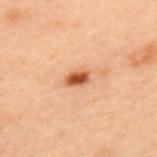Recorded during total-body skin imaging; not selected for excision or biopsy. The subject is a male aged approximately 55. Approximately 2.5 mm at its widest. A 15 mm crop from a total-body photograph taken for skin-cancer surveillance. This is a cross-polarized tile. On the upper back.